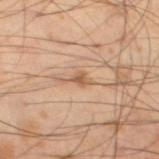{"image": {"source": "total-body photography crop", "field_of_view_mm": 15}, "automated_metrics": {"border_irregularity_0_10": 4.5, "color_variation_0_10": 1.0, "peripheral_color_asymmetry": 0.5}, "patient": {"sex": "male", "age_approx": 55}, "lesion_size": {"long_diameter_mm_approx": 2.5}, "site": "left lower leg", "lighting": "cross-polarized"}